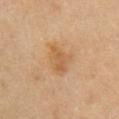Impression:
The lesion was tiled from a total-body skin photograph and was not biopsied.
Clinical summary:
A 15 mm crop from a total-body photograph taken for skin-cancer surveillance. From the arm. The subject is a female aged 58 to 62.